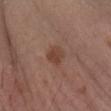<tbp_lesion>
<biopsy_status>not biopsied; imaged during a skin examination</biopsy_status>
<automated_metrics>
  <area_mm2_approx>5.0</area_mm2_approx>
  <eccentricity>0.6</eccentricity>
  <shape_asymmetry>0.3</shape_asymmetry>
  <nevus_likeness_0_100>75</nevus_likeness_0_100>
</automated_metrics>
<image>
  <source>total-body photography crop</source>
  <field_of_view_mm>15</field_of_view_mm>
</image>
<lesion_size>
  <long_diameter_mm_approx>2.5</long_diameter_mm_approx>
</lesion_size>
<patient>
  <sex>male</sex>
  <age_approx>70</age_approx>
</patient>
<site>right forearm</site>
</tbp_lesion>